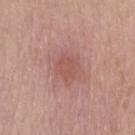<case>
  <biopsy_status>not biopsied; imaged during a skin examination</biopsy_status>
</case>